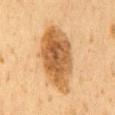Q: Was a biopsy performed?
A: imaged on a skin check; not biopsied
Q: How was this image acquired?
A: ~15 mm crop, total-body skin-cancer survey
Q: How large is the lesion?
A: about 9 mm
Q: What are the patient's age and sex?
A: male, approximately 60 years of age
Q: Illumination type?
A: cross-polarized illumination
Q: What is the anatomic site?
A: the back